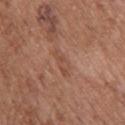workup: total-body-photography surveillance lesion; no biopsy
acquisition: ~15 mm tile from a whole-body skin photo
anatomic site: the upper back
automated lesion analysis: a nevus-likeness score of about 0/100
patient: male, in their mid- to late 70s
illumination: white-light illumination